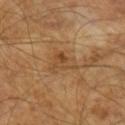Clinical impression:
This lesion was catalogued during total-body skin photography and was not selected for biopsy.
Acquisition and patient details:
A 15 mm crop from a total-body photograph taken for skin-cancer surveillance. A male patient approximately 65 years of age. The recorded lesion diameter is about 4 mm.